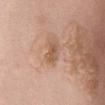Findings:
• image · ~15 mm crop, total-body skin-cancer survey
• body site · the abdomen
• automated metrics · a border-irregularity index near 2/10, a color-variation rating of about 2.5/10, and a peripheral color-asymmetry measure near 1
• illumination · white-light
• subject · female, aged 63–67
• size · ≈2.5 mm